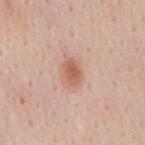Recorded during total-body skin imaging; not selected for excision or biopsy. A male patient in their mid-50s. The lesion-visualizer software estimated a footprint of about 5 mm² and an eccentricity of roughly 0.75. The software also gave a lesion-to-skin contrast of about 7.5 (normalized; higher = more distinct). The software also gave a border-irregularity index near 1.5/10, a color-variation rating of about 3/10, and a peripheral color-asymmetry measure near 1. Approximately 3 mm at its widest. Located on the mid back. Imaged with white-light lighting. This image is a 15 mm lesion crop taken from a total-body photograph.